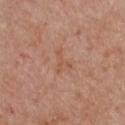biopsy status: catalogued during a skin exam; not biopsied
illumination: white-light illumination
acquisition: ~15 mm tile from a whole-body skin photo
subject: female, in their mid-50s
lesion size: about 3 mm
site: the chest